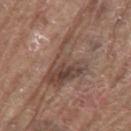Impression: Captured during whole-body skin photography for melanoma surveillance; the lesion was not biopsied. Background: Measured at roughly 7.5 mm in maximum diameter. The lesion is located on the right upper arm. Cropped from a whole-body photographic skin survey; the tile spans about 15 mm. A male patient roughly 80 years of age. The tile uses white-light illumination. Automated tile analysis of the lesion measured a border-irregularity index near 8/10. And it measured a classifier nevus-likeness of about 0/100 and a lesion-detection confidence of about 75/100.